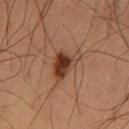Q: Was a biopsy performed?
A: total-body-photography surveillance lesion; no biopsy
Q: How was the tile lit?
A: cross-polarized
Q: What is the lesion's diameter?
A: ≈4 mm
Q: Patient demographics?
A: male, aged 58 to 62
Q: What did automated image analysis measure?
A: a lesion area of about 8.5 mm², a shape eccentricity near 0.65, and a symmetry-axis asymmetry near 0.45; an average lesion color of about L≈39 a*≈21 b*≈30 (CIELAB), roughly 13 lightness units darker than nearby skin, and a lesion-to-skin contrast of about 10 (normalized; higher = more distinct); a border-irregularity rating of about 4.5/10 and a within-lesion color-variation index near 6.5/10; an automated nevus-likeness rating near 95 out of 100 and a detector confidence of about 100 out of 100 that the crop contains a lesion
Q: Lesion location?
A: the left thigh
Q: What is the imaging modality?
A: ~15 mm crop, total-body skin-cancer survey